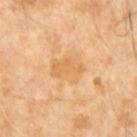{"biopsy_status": "not biopsied; imaged during a skin examination", "image": {"source": "total-body photography crop", "field_of_view_mm": 15}, "lighting": "cross-polarized", "automated_metrics": {"area_mm2_approx": 10.0, "shape_asymmetry": 0.3, "border_irregularity_0_10": 3.0, "color_variation_0_10": 2.5, "peripheral_color_asymmetry": 1.0, "lesion_detection_confidence_0_100": 100}, "patient": {"sex": "male", "age_approx": 65}}